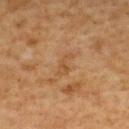The lesion was photographed on a routine skin check and not biopsied; there is no pathology result.
The subject is a male approximately 60 years of age.
About 3 mm across.
The tile uses cross-polarized illumination.
On the upper back.
This image is a 15 mm lesion crop taken from a total-body photograph.
The lesion-visualizer software estimated a lesion area of about 3 mm² and two-axis asymmetry of about 0.6. The analysis additionally found a border-irregularity index near 6/10 and radial color variation of about 0.5.